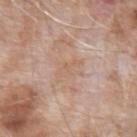Recorded during total-body skin imaging; not selected for excision or biopsy.
The lesion is located on the left upper arm.
Approximately 3 mm at its widest.
An algorithmic analysis of the crop reported an eccentricity of roughly 0.9. It also reported a mean CIELAB color near L≈61 a*≈18 b*≈30, roughly 5 lightness units darker than nearby skin, and a normalized border contrast of about 4.5. It also reported a nevus-likeness score of about 0/100 and a detector confidence of about 100 out of 100 that the crop contains a lesion.
This is a white-light tile.
A male subject approximately 75 years of age.
A roughly 15 mm field-of-view crop from a total-body skin photograph.